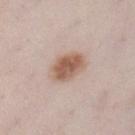workup=no biopsy performed (imaged during a skin exam); anatomic site=the left lower leg; subject=female, aged 28 to 32; image=~15 mm tile from a whole-body skin photo; size=about 4.5 mm.